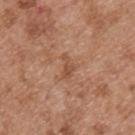No biopsy was performed on this lesion — it was imaged during a full skin examination and was not determined to be concerning.
On the upper back.
A lesion tile, about 15 mm wide, cut from a 3D total-body photograph.
A male patient in their mid-50s.